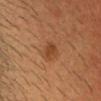patient:
  sex: female
  age_approx: 50
image:
  source: total-body photography crop
  field_of_view_mm: 15